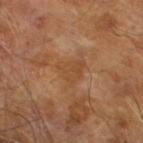Part of a total-body skin-imaging series; this lesion was reviewed on a skin check and was not flagged for biopsy.
An algorithmic analysis of the crop reported a nevus-likeness score of about 0/100 and a detector confidence of about 100 out of 100 that the crop contains a lesion.
Imaged with cross-polarized lighting.
Longest diameter approximately 3.5 mm.
A 15 mm crop from a total-body photograph taken for skin-cancer surveillance.
A male patient, about 65 years old.